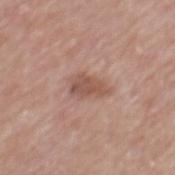follow-up: imaged on a skin check; not biopsied
acquisition: ~15 mm tile from a whole-body skin photo
illumination: white-light illumination
anatomic site: the mid back
lesion size: about 3.5 mm
TBP lesion metrics: an area of roughly 6 mm²; about 10 CIELAB-L* units darker than the surrounding skin and a normalized border contrast of about 7; a border-irregularity index near 3.5/10, a color-variation rating of about 3.5/10, and a peripheral color-asymmetry measure near 1; lesion-presence confidence of about 100/100
patient: male, aged around 70